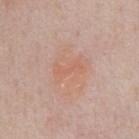This lesion was catalogued during total-body skin photography and was not selected for biopsy.
The subject is a male aged around 60.
A lesion tile, about 15 mm wide, cut from a 3D total-body photograph.
The recorded lesion diameter is about 3.5 mm.
The tile uses white-light illumination.
The lesion is located on the chest.